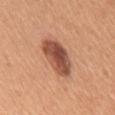{"biopsy_status": "not biopsied; imaged during a skin examination", "image": {"source": "total-body photography crop", "field_of_view_mm": 15}, "lesion_size": {"long_diameter_mm_approx": 5.5}, "lighting": "white-light", "patient": {"sex": "female", "age_approx": 55}, "site": "upper back"}